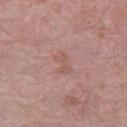<tbp_lesion>
<biopsy_status>not biopsied; imaged during a skin examination</biopsy_status>
<patient>
  <sex>female</sex>
  <age_approx>55</age_approx>
</patient>
<lesion_size>
  <long_diameter_mm_approx>3.0</long_diameter_mm_approx>
</lesion_size>
<image>
  <source>total-body photography crop</source>
  <field_of_view_mm>15</field_of_view_mm>
</image>
<site>right thigh</site>
<automated_metrics>
  <cielab_L>55</cielab_L>
  <cielab_a>22</cielab_a>
  <cielab_b>24</cielab_b>
  <vs_skin_darker_L>6.0</vs_skin_darker_L>
  <border_irregularity_0_10>3.5</border_irregularity_0_10>
  <color_variation_0_10>2.0</color_variation_0_10>
  <peripheral_color_asymmetry>0.5</peripheral_color_asymmetry>
</automated_metrics>
</tbp_lesion>